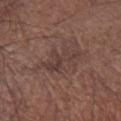biopsy status = catalogued during a skin exam; not biopsied
anatomic site = the left forearm
subject = male, aged approximately 65
size = ~4.5 mm (longest diameter)
imaging modality = 15 mm crop, total-body photography
lighting = white-light illumination